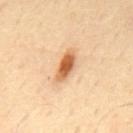<lesion>
  <biopsy_status>not biopsied; imaged during a skin examination</biopsy_status>
  <lighting>cross-polarized</lighting>
  <automated_metrics>
    <cielab_L>59</cielab_L>
    <cielab_a>23</cielab_a>
    <cielab_b>39</cielab_b>
    <vs_skin_darker_L>16.0</vs_skin_darker_L>
    <vs_skin_contrast_norm>10.5</vs_skin_contrast_norm>
    <nevus_likeness_0_100>100</nevus_likeness_0_100>
  </automated_metrics>
  <patient>
    <sex>male</sex>
    <age_approx>65</age_approx>
  </patient>
  <site>upper back</site>
  <lesion_size>
    <long_diameter_mm_approx>4.0</long_diameter_mm_approx>
  </lesion_size>
  <image>
    <source>total-body photography crop</source>
    <field_of_view_mm>15</field_of_view_mm>
  </image>
</lesion>